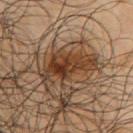This lesion was catalogued during total-body skin photography and was not selected for biopsy. From the right upper arm. This is a cross-polarized tile. A male subject aged around 65. Cropped from a total-body skin-imaging series; the visible field is about 15 mm. The recorded lesion diameter is about 7 mm.